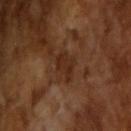| feature | finding |
|---|---|
| notes | catalogued during a skin exam; not biopsied |
| lesion diameter | ~3 mm (longest diameter) |
| image | ~15 mm crop, total-body skin-cancer survey |
| patient | male, in their mid-60s |
| image-analysis metrics | a shape eccentricity near 0.8; a mean CIELAB color near L≈26 a*≈19 b*≈28, a lesion–skin lightness drop of about 7, and a lesion-to-skin contrast of about 7.5 (normalized; higher = more distinct); internal color variation of about 1 on a 0–10 scale and radial color variation of about 0.5; a nevus-likeness score of about 0/100 |
| lighting | cross-polarized illumination |